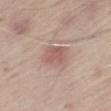Findings:
* imaging modality — ~15 mm crop, total-body skin-cancer survey
* site — the right thigh
* subject — male, about 65 years old